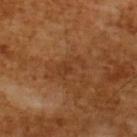– patient: male, approximately 65 years of age
– diameter: ≈4 mm
– illumination: cross-polarized illumination
– imaging modality: ~15 mm tile from a whole-body skin photo